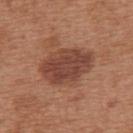{"biopsy_status": "not biopsied; imaged during a skin examination", "patient": {"sex": "female", "age_approx": 40}, "lesion_size": {"long_diameter_mm_approx": 6.5}, "lighting": "white-light", "site": "upper back", "image": {"source": "total-body photography crop", "field_of_view_mm": 15}}